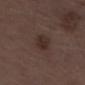notes: imaged on a skin check; not biopsied
body site: the left thigh
imaging modality: total-body-photography crop, ~15 mm field of view
patient: male, roughly 70 years of age